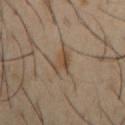Case summary:
– diameter — ≈2.5 mm
– tile lighting — cross-polarized illumination
– patient — male, aged 38 to 42
– image source — 15 mm crop, total-body photography
– TBP lesion metrics — a lesion area of about 4 mm²; a mean CIELAB color near L≈45 a*≈14 b*≈29 and a lesion–skin lightness drop of about 8; a border-irregularity index near 4.5/10 and a color-variation rating of about 3.5/10; a classifier nevus-likeness of about 10/100 and lesion-presence confidence of about 100/100
– site — the right upper arm